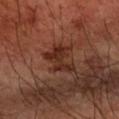Located on the arm. This image is a 15 mm lesion crop taken from a total-body photograph. The tile uses cross-polarized illumination. A male subject, aged around 60.